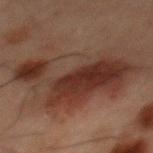Q: Is there a histopathology result?
A: catalogued during a skin exam; not biopsied
Q: Automated lesion metrics?
A: a lesion color around L≈25 a*≈16 b*≈20 in CIELAB and a normalized lesion–skin contrast near 9; border irregularity of about 5 on a 0–10 scale and a peripheral color-asymmetry measure near 2.5
Q: What is the lesion's diameter?
A: ~10.5 mm (longest diameter)
Q: What is the anatomic site?
A: the mid back
Q: How was this image acquired?
A: total-body-photography crop, ~15 mm field of view
Q: Patient demographics?
A: male, aged 58–62